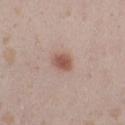Assessment: No biopsy was performed on this lesion — it was imaged during a full skin examination and was not determined to be concerning. Clinical summary: Captured under white-light illumination. A 15 mm crop from a total-body photograph taken for skin-cancer surveillance. The lesion is on the left thigh. The patient is a female aged approximately 25.